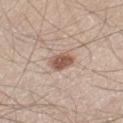biopsy status — imaged on a skin check; not biopsied | patient — male, aged 58 to 62 | lighting — white-light illumination | diameter — ~2.5 mm (longest diameter) | image source — 15 mm crop, total-body photography | location — the left thigh.